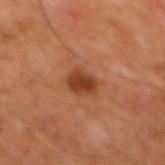workup: no biopsy performed (imaged during a skin exam); anatomic site: the chest; tile lighting: cross-polarized; lesion diameter: ≈3 mm; patient: male, aged 58–62; acquisition: total-body-photography crop, ~15 mm field of view.